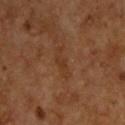Acquisition and patient details: Imaged with cross-polarized lighting. The recorded lesion diameter is about 4 mm. From the back. A male patient, about 60 years old. A close-up tile cropped from a whole-body skin photograph, about 15 mm across.